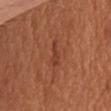Recorded during total-body skin imaging; not selected for excision or biopsy. A 15 mm close-up tile from a total-body photography series done for melanoma screening. The lesion is on the chest. A male patient, aged 63 to 67. The tile uses white-light illumination. About 3.5 mm across.